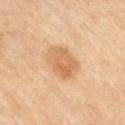Q: Was this lesion biopsied?
A: imaged on a skin check; not biopsied
Q: How was this image acquired?
A: ~15 mm tile from a whole-body skin photo
Q: What lighting was used for the tile?
A: cross-polarized illumination
Q: What are the patient's age and sex?
A: female, roughly 60 years of age
Q: How large is the lesion?
A: about 4.5 mm
Q: Lesion location?
A: the leg